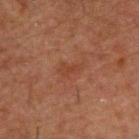workup: imaged on a skin check; not biopsied
image: ~15 mm tile from a whole-body skin photo
subject: male, in their 50s
anatomic site: the back
lighting: cross-polarized illumination
lesion size: ~2.5 mm (longest diameter)
automated metrics: a lesion area of about 3 mm² and two-axis asymmetry of about 0.45; an average lesion color of about L≈32 a*≈20 b*≈25 (CIELAB), roughly 4 lightness units darker than nearby skin, and a normalized border contrast of about 4.5; a border-irregularity rating of about 4/10 and a within-lesion color-variation index near 0.5/10